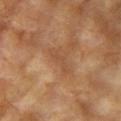Impression: No biopsy was performed on this lesion — it was imaged during a full skin examination and was not determined to be concerning. Context: From the arm. The subject is a female aged around 75. A close-up tile cropped from a whole-body skin photograph, about 15 mm across. The lesion's longest dimension is about 3.5 mm. Captured under cross-polarized illumination.